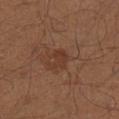Q: Is there a histopathology result?
A: imaged on a skin check; not biopsied
Q: How large is the lesion?
A: ≈2.5 mm
Q: What kind of image is this?
A: total-body-photography crop, ~15 mm field of view
Q: Lesion location?
A: the left lower leg
Q: Patient demographics?
A: male, aged around 40
Q: What lighting was used for the tile?
A: cross-polarized illumination